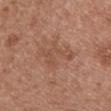The lesion was photographed on a routine skin check and not biopsied; there is no pathology result. Imaged with white-light lighting. A 15 mm close-up extracted from a 3D total-body photography capture. Approximately 5 mm at its widest. Located on the chest. The subject is a female about 40 years old.